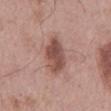Part of a total-body skin-imaging series; this lesion was reviewed on a skin check and was not flagged for biopsy. Longest diameter approximately 5.5 mm. A 15 mm crop from a total-body photograph taken for skin-cancer surveillance. This is a white-light tile. The lesion is located on the mid back. A male patient, aged 53–57. Automated tile analysis of the lesion measured a lesion area of about 11 mm², an outline eccentricity of about 0.9 (0 = round, 1 = elongated), and a symmetry-axis asymmetry near 0.2. And it measured a normalized border contrast of about 9. The analysis additionally found a within-lesion color-variation index near 4/10 and peripheral color asymmetry of about 1.5. The analysis additionally found an automated nevus-likeness rating near 75 out of 100 and a detector confidence of about 100 out of 100 that the crop contains a lesion.